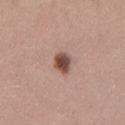follow-up: total-body-photography surveillance lesion; no biopsy
anatomic site: the left thigh
automated metrics: a footprint of about 5 mm², an outline eccentricity of about 0.55 (0 = round, 1 = elongated), and a symmetry-axis asymmetry near 0.15; a lesion color around L≈48 a*≈19 b*≈24 in CIELAB and a normalized lesion–skin contrast near 11; border irregularity of about 1.5 on a 0–10 scale and a within-lesion color-variation index near 4/10
acquisition: ~15 mm tile from a whole-body skin photo
subject: female, aged 23 to 27
tile lighting: white-light
lesion diameter: ≈2.5 mm